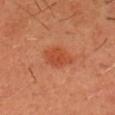<record>
<biopsy_status>not biopsied; imaged during a skin examination</biopsy_status>
<patient>
  <sex>male</sex>
  <age_approx>40</age_approx>
</patient>
<site>head or neck</site>
<automated_metrics>
  <vs_skin_darker_L>8.0</vs_skin_darker_L>
  <vs_skin_contrast_norm>6.5</vs_skin_contrast_norm>
</automated_metrics>
<lesion_size>
  <long_diameter_mm_approx>4.0</long_diameter_mm_approx>
</lesion_size>
<image>
  <source>total-body photography crop</source>
  <field_of_view_mm>15</field_of_view_mm>
</image>
</record>